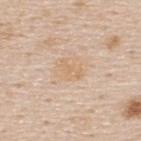follow-up = catalogued during a skin exam; not biopsied
TBP lesion metrics = a lesion-detection confidence of about 100/100
tile lighting = white-light illumination
site = the upper back
imaging modality = ~15 mm tile from a whole-body skin photo
subject = male, about 45 years old